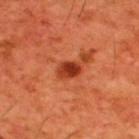The lesion was tiled from a total-body skin photograph and was not biopsied. On the upper back. This is a cross-polarized tile. A male subject, aged 58 to 62. Approximately 2.5 mm at its widest. A lesion tile, about 15 mm wide, cut from a 3D total-body photograph.